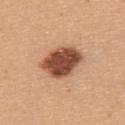Captured during whole-body skin photography for melanoma surveillance; the lesion was not biopsied. Automated image analysis of the tile measured a lesion color around L≈50 a*≈24 b*≈32 in CIELAB and a lesion-to-skin contrast of about 13 (normalized; higher = more distinct). And it measured a border-irregularity rating of about 1.5/10 and a peripheral color-asymmetry measure near 1.5. The recorded lesion diameter is about 5.5 mm. The lesion is located on the upper back. The subject is a female roughly 45 years of age. A 15 mm crop from a total-body photograph taken for skin-cancer surveillance. The tile uses white-light illumination.